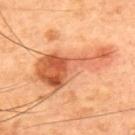<case>
<biopsy_status>not biopsied; imaged during a skin examination</biopsy_status>
<lesion_size>
  <long_diameter_mm_approx>10.0</long_diameter_mm_approx>
</lesion_size>
<image>
  <source>total-body photography crop</source>
  <field_of_view_mm>15</field_of_view_mm>
</image>
<patient>
  <sex>male</sex>
  <age_approx>60</age_approx>
</patient>
<lighting>cross-polarized</lighting>
<site>upper back</site>
</case>